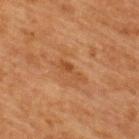* biopsy status: imaged on a skin check; not biopsied
* anatomic site: the upper back
* illumination: cross-polarized
* size: ≈3 mm
* image source: 15 mm crop, total-body photography
* patient: female, about 40 years old